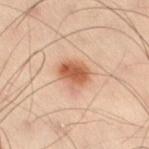The lesion was photographed on a routine skin check and not biopsied; there is no pathology result. This image is a 15 mm lesion crop taken from a total-body photograph. Measured at roughly 3.5 mm in maximum diameter. This is a cross-polarized tile. A male patient, about 50 years old. On the left leg.